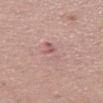follow-up=no biopsy performed (imaged during a skin exam)
image=total-body-photography crop, ~15 mm field of view
automated lesion analysis=a within-lesion color-variation index near 1.5/10 and radial color variation of about 0.5; an automated nevus-likeness rating near 0 out of 100 and lesion-presence confidence of about 100/100
subject=male, aged 53 to 57
lesion size=about 3.5 mm
body site=the right thigh